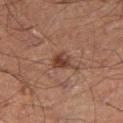Q: What kind of image is this?
A: 15 mm crop, total-body photography
Q: Automated lesion metrics?
A: an outline eccentricity of about 0.6 (0 = round, 1 = elongated) and two-axis asymmetry of about 0.3; a border-irregularity rating of about 2.5/10, a within-lesion color-variation index near 4.5/10, and a peripheral color-asymmetry measure near 1.5; an automated nevus-likeness rating near 90 out of 100 and lesion-presence confidence of about 100/100
Q: Where on the body is the lesion?
A: the right thigh
Q: What lighting was used for the tile?
A: cross-polarized illumination
Q: What are the patient's age and sex?
A: male, aged 58–62
Q: What is the lesion's diameter?
A: ≈2.5 mm